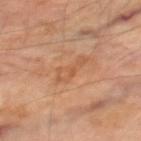Part of a total-body skin-imaging series; this lesion was reviewed on a skin check and was not flagged for biopsy.
A lesion tile, about 15 mm wide, cut from a 3D total-body photograph.
A male patient, aged approximately 70.
On the right thigh.
This is a cross-polarized tile.
About 4 mm across.